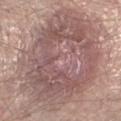notes: catalogued during a skin exam; not biopsied
image source: total-body-photography crop, ~15 mm field of view
subject: male, in their 40s
body site: the left lower leg
automated lesion analysis: an eccentricity of roughly 0.6 and two-axis asymmetry of about 0.15; a lesion color around L≈55 a*≈18 b*≈20 in CIELAB; border irregularity of about 2.5 on a 0–10 scale, internal color variation of about 6.5 on a 0–10 scale, and a peripheral color-asymmetry measure near 2.5
illumination: white-light